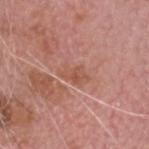This lesion was catalogued during total-body skin photography and was not selected for biopsy.
An algorithmic analysis of the crop reported about 7 CIELAB-L* units darker than the surrounding skin and a normalized border contrast of about 6.
Located on the head or neck.
The lesion's longest dimension is about 3.5 mm.
A male subject in their mid- to late 70s.
A 15 mm close-up extracted from a 3D total-body photography capture.